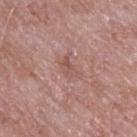follow-up — total-body-photography surveillance lesion; no biopsy | subject — male, in their mid- to late 60s | image — total-body-photography crop, ~15 mm field of view | site — the upper back.